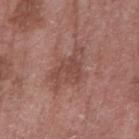Part of a total-body skin-imaging series; this lesion was reviewed on a skin check and was not flagged for biopsy. This is a white-light tile. Cropped from a whole-body photographic skin survey; the tile spans about 15 mm. The patient is a male aged approximately 75. On the right forearm.